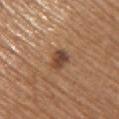Q: Was this lesion biopsied?
A: total-body-photography surveillance lesion; no biopsy
Q: What is the imaging modality?
A: total-body-photography crop, ~15 mm field of view
Q: What are the patient's age and sex?
A: male, in their mid- to late 60s
Q: What is the anatomic site?
A: the upper back
Q: How was the tile lit?
A: white-light illumination
Q: Automated lesion metrics?
A: an area of roughly 5 mm², an eccentricity of roughly 0.5, and two-axis asymmetry of about 0.25
Q: What is the lesion's diameter?
A: ~2.5 mm (longest diameter)